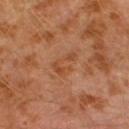Impression:
The lesion was photographed on a routine skin check and not biopsied; there is no pathology result.
Background:
Approximately 3.5 mm at its widest. The total-body-photography lesion software estimated a lesion area of about 5.5 mm², an outline eccentricity of about 0.8 (0 = round, 1 = elongated), and a shape-asymmetry score of about 0.5 (0 = symmetric). It also reported an automated nevus-likeness rating near 0 out of 100. The lesion is located on the left lower leg. A male patient aged around 30. Cropped from a whole-body photographic skin survey; the tile spans about 15 mm.